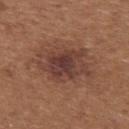<case>
  <biopsy_status>not biopsied; imaged during a skin examination</biopsy_status>
</case>